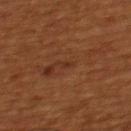Assessment: Recorded during total-body skin imaging; not selected for excision or biopsy. Background: The subject is a male in their mid-40s. This is a cross-polarized tile. About 1 mm across. On the upper back. A 15 mm crop from a total-body photograph taken for skin-cancer surveillance.